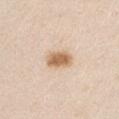notes: catalogued during a skin exam; not biopsied | size: ~3.5 mm (longest diameter) | body site: the right upper arm | lighting: white-light | patient: male, aged approximately 45 | acquisition: total-body-photography crop, ~15 mm field of view.